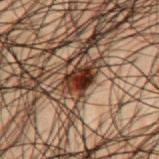| field | value |
|---|---|
| biopsy status | total-body-photography surveillance lesion; no biopsy |
| subject | male, aged approximately 50 |
| site | the mid back |
| image | 15 mm crop, total-body photography |